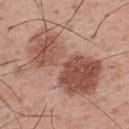Impression:
Captured during whole-body skin photography for melanoma surveillance; the lesion was not biopsied.
Image and clinical context:
A male subject roughly 65 years of age. From the upper back. Cropped from a total-body skin-imaging series; the visible field is about 15 mm. The total-body-photography lesion software estimated a shape eccentricity near 0.85 and two-axis asymmetry of about 0.35. The analysis additionally found border irregularity of about 7 on a 0–10 scale, internal color variation of about 7.5 on a 0–10 scale, and peripheral color asymmetry of about 3.